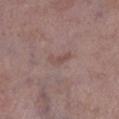follow-up: imaged on a skin check; not biopsied | subject: male, aged 73 to 77 | location: the right lower leg | illumination: white-light | automated metrics: a footprint of about 3 mm², a shape eccentricity near 0.9, and a shape-asymmetry score of about 0.5 (0 = symmetric); a mean CIELAB color near L≈49 a*≈18 b*≈21, roughly 6 lightness units darker than nearby skin, and a lesion-to-skin contrast of about 5 (normalized; higher = more distinct); border irregularity of about 4.5 on a 0–10 scale, a color-variation rating of about 0/10, and a peripheral color-asymmetry measure near 0; an automated nevus-likeness rating near 0 out of 100 and a detector confidence of about 100 out of 100 that the crop contains a lesion | imaging modality: ~15 mm tile from a whole-body skin photo.